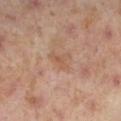Q: Was a biopsy performed?
A: no biopsy performed (imaged during a skin exam)
Q: Where on the body is the lesion?
A: the left lower leg
Q: Illumination type?
A: cross-polarized
Q: What kind of image is this?
A: total-body-photography crop, ~15 mm field of view
Q: How large is the lesion?
A: ~3 mm (longest diameter)
Q: What are the patient's age and sex?
A: female, aged 38 to 42